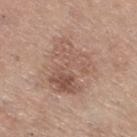Acquisition and patient details:
Automated tile analysis of the lesion measured a lesion color around L≈55 a*≈18 b*≈26 in CIELAB and a normalized lesion–skin contrast near 6. And it measured a border-irregularity index near 4/10, internal color variation of about 6.5 on a 0–10 scale, and peripheral color asymmetry of about 2.5. And it measured an automated nevus-likeness rating near 5 out of 100 and a lesion-detection confidence of about 100/100. A female patient in their mid-60s. Cropped from a whole-body photographic skin survey; the tile spans about 15 mm. This is a white-light tile. On the right thigh.